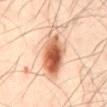follow-up = total-body-photography surveillance lesion; no biopsy
anatomic site = the back
image = total-body-photography crop, ~15 mm field of view
lighting = cross-polarized
patient = male, approximately 50 years of age
lesion diameter = about 8 mm